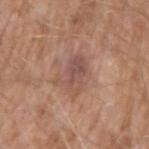The lesion was photographed on a routine skin check and not biopsied; there is no pathology result.
A roughly 15 mm field-of-view crop from a total-body skin photograph.
On the right upper arm.
The lesion's longest dimension is about 4 mm.
This is a white-light tile.
A male subject about 60 years old.